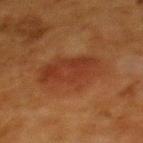Assessment: No biopsy was performed on this lesion — it was imaged during a full skin examination and was not determined to be concerning. Image and clinical context: The patient is a female aged 48–52. The recorded lesion diameter is about 6.5 mm. A 15 mm close-up tile from a total-body photography series done for melanoma screening. The lesion is on the upper back. Imaged with cross-polarized lighting.